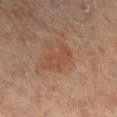Captured during whole-body skin photography for melanoma surveillance; the lesion was not biopsied. A female patient, about 80 years old. Measured at roughly 4 mm in maximum diameter. The lesion-visualizer software estimated a lesion area of about 5 mm², an eccentricity of roughly 0.9, and a shape-asymmetry score of about 0.4 (0 = symmetric). And it measured a lesion color around L≈40 a*≈20 b*≈27 in CIELAB, about 5 CIELAB-L* units darker than the surrounding skin, and a normalized lesion–skin contrast near 5. The software also gave a lesion-detection confidence of about 100/100. A lesion tile, about 15 mm wide, cut from a 3D total-body photograph. Located on the right lower leg.